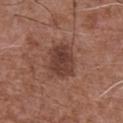The lesion's longest dimension is about 4 mm. An algorithmic analysis of the crop reported an area of roughly 11 mm² and an eccentricity of roughly 0.6. The software also gave a mean CIELAB color near L≈39 a*≈21 b*≈24, about 10 CIELAB-L* units darker than the surrounding skin, and a lesion-to-skin contrast of about 8.5 (normalized; higher = more distinct). A male subject in their mid-70s. The tile uses white-light illumination. A lesion tile, about 15 mm wide, cut from a 3D total-body photograph. On the chest.